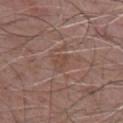Part of a total-body skin-imaging series; this lesion was reviewed on a skin check and was not flagged for biopsy.
Automated tile analysis of the lesion measured a lesion color around L≈46 a*≈18 b*≈25 in CIELAB, roughly 5 lightness units darker than nearby skin, and a normalized lesion–skin contrast near 5. And it measured a border-irregularity rating of about 3/10 and internal color variation of about 1.5 on a 0–10 scale.
A 15 mm crop from a total-body photograph taken for skin-cancer surveillance.
On the chest.
About 2.5 mm across.
A male patient, roughly 70 years of age.
Captured under white-light illumination.